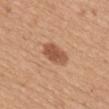The lesion was tiled from a total-body skin photograph and was not biopsied. The lesion is on the mid back. A 15 mm close-up tile from a total-body photography series done for melanoma screening. A female subject aged around 40.